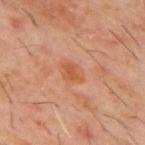Part of a total-body skin-imaging series; this lesion was reviewed on a skin check and was not flagged for biopsy. Approximately 3 mm at its widest. The patient is a male approximately 60 years of age. A roughly 15 mm field-of-view crop from a total-body skin photograph. The lesion is on the mid back. Automated tile analysis of the lesion measured a border-irregularity index near 2.5/10, internal color variation of about 2 on a 0–10 scale, and radial color variation of about 0.5. The software also gave a nevus-likeness score of about 20/100 and lesion-presence confidence of about 100/100. This is a cross-polarized tile.